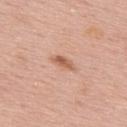Part of a total-body skin-imaging series; this lesion was reviewed on a skin check and was not flagged for biopsy.
A male subject aged 53 to 57.
This image is a 15 mm lesion crop taken from a total-body photograph.
On the upper back.
The lesion-visualizer software estimated an area of roughly 3.5 mm², an outline eccentricity of about 0.9 (0 = round, 1 = elongated), and a shape-asymmetry score of about 0.3 (0 = symmetric). And it measured an average lesion color of about L≈60 a*≈23 b*≈32 (CIELAB) and a lesion–skin lightness drop of about 11. And it measured a detector confidence of about 100 out of 100 that the crop contains a lesion.
The tile uses white-light illumination.